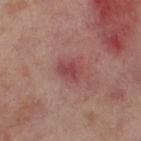follow-up = total-body-photography surveillance lesion; no biopsy | site = the right thigh | lighting = cross-polarized | subject = female, approximately 55 years of age | acquisition = 15 mm crop, total-body photography | lesion size = ~3 mm (longest diameter).